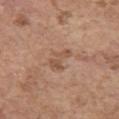Assessment:
Imaged during a routine full-body skin examination; the lesion was not biopsied and no histopathology is available.
Background:
The lesion-visualizer software estimated a lesion area of about 4.5 mm², a shape eccentricity near 0.9, and a shape-asymmetry score of about 0.5 (0 = symmetric). The analysis additionally found a mean CIELAB color near L≈52 a*≈20 b*≈31 and a lesion–skin lightness drop of about 8. And it measured a border-irregularity rating of about 6/10, a within-lesion color-variation index near 1/10, and radial color variation of about 0.5. A female subject roughly 65 years of age. From the left upper arm. A region of skin cropped from a whole-body photographic capture, roughly 15 mm wide. Imaged with white-light lighting. The recorded lesion diameter is about 3.5 mm.